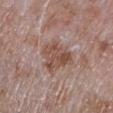{"biopsy_status": "not biopsied; imaged during a skin examination", "site": "arm", "patient": {"sex": "male", "age_approx": 65}, "image": {"source": "total-body photography crop", "field_of_view_mm": 15}}